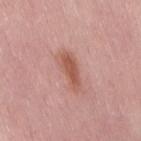Captured during whole-body skin photography for melanoma surveillance; the lesion was not biopsied.
Located on the right thigh.
This is a white-light tile.
The patient is a female aged around 50.
Cropped from a total-body skin-imaging series; the visible field is about 15 mm.
The total-body-photography lesion software estimated an area of roughly 7 mm², an eccentricity of roughly 0.9, and a symmetry-axis asymmetry near 0.25. It also reported an automated nevus-likeness rating near 70 out of 100 and a detector confidence of about 100 out of 100 that the crop contains a lesion.